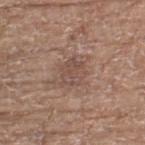The lesion was tiled from a total-body skin photograph and was not biopsied. A female patient aged around 75. Cropped from a whole-body photographic skin survey; the tile spans about 15 mm. On the left thigh.